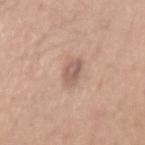Impression: Imaged during a routine full-body skin examination; the lesion was not biopsied and no histopathology is available. Background: The tile uses white-light illumination. Cropped from a whole-body photographic skin survey; the tile spans about 15 mm. A male subject aged 28 to 32. An algorithmic analysis of the crop reported a lesion color around L≈58 a*≈18 b*≈25 in CIELAB, about 10 CIELAB-L* units darker than the surrounding skin, and a normalized lesion–skin contrast near 6.5. The software also gave a lesion-detection confidence of about 100/100. Approximately 3 mm at its widest. The lesion is located on the back.